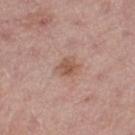workup: total-body-photography surveillance lesion; no biopsy | body site: the right thigh | subject: male, aged 53 to 57 | image source: total-body-photography crop, ~15 mm field of view | automated lesion analysis: a lesion-to-skin contrast of about 7 (normalized; higher = more distinct); lesion-presence confidence of about 100/100 | lesion diameter: about 3 mm | lighting: white-light illumination.